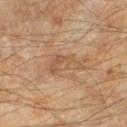{
  "biopsy_status": "not biopsied; imaged during a skin examination",
  "patient": {
    "age_approx": 55
  },
  "lighting": "cross-polarized",
  "image": {
    "source": "total-body photography crop",
    "field_of_view_mm": 15
  },
  "site": "left lower leg"
}